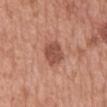* biopsy status — imaged on a skin check; not biopsied
* anatomic site — the mid back
* lesion diameter — about 4 mm
* patient — male, approximately 70 years of age
* tile lighting — white-light illumination
* image source — 15 mm crop, total-body photography